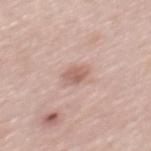This lesion was catalogued during total-body skin photography and was not selected for biopsy.
Longest diameter approximately 2.5 mm.
A close-up tile cropped from a whole-body skin photograph, about 15 mm across.
A male subject, approximately 55 years of age.
From the mid back.
The tile uses white-light illumination.